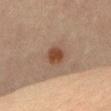{
  "biopsy_status": "not biopsied; imaged during a skin examination",
  "automated_metrics": {
    "area_mm2_approx": 4.5,
    "eccentricity": 0.6,
    "shape_asymmetry": 0.25,
    "cielab_L": 36,
    "cielab_a": 18,
    "cielab_b": 27
  },
  "image": {
    "source": "total-body photography crop",
    "field_of_view_mm": 15
  },
  "lighting": "cross-polarized",
  "site": "abdomen",
  "patient": {
    "sex": "male",
    "age_approx": 60
  },
  "lesion_size": {
    "long_diameter_mm_approx": 2.5
  }
}